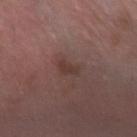site = the left forearm | tile lighting = white-light | diameter = ~3 mm (longest diameter) | subject = male, roughly 60 years of age | image source = ~15 mm tile from a whole-body skin photo.